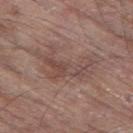Captured during whole-body skin photography for melanoma surveillance; the lesion was not biopsied.
The tile uses white-light illumination.
On the left thigh.
A roughly 15 mm field-of-view crop from a total-body skin photograph.
An algorithmic analysis of the crop reported an outline eccentricity of about 0.85 (0 = round, 1 = elongated). The analysis additionally found a border-irregularity index near 9.5/10, a within-lesion color-variation index near 3.5/10, and a peripheral color-asymmetry measure near 1.
The subject is a male aged 78 to 82.